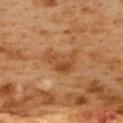{
  "biopsy_status": "not biopsied; imaged during a skin examination"
}